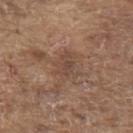<tbp_lesion>
<biopsy_status>not biopsied; imaged during a skin examination</biopsy_status>
<site>upper back</site>
<automated_metrics>
  <border_irregularity_0_10>5.0</border_irregularity_0_10>
  <color_variation_0_10>2.5</color_variation_0_10>
  <peripheral_color_asymmetry>0.5</peripheral_color_asymmetry>
</automated_metrics>
<lesion_size>
  <long_diameter_mm_approx>3.5</long_diameter_mm_approx>
</lesion_size>
<patient>
  <sex>male</sex>
  <age_approx>80</age_approx>
</patient>
<lighting>white-light</lighting>
<image>
  <source>total-body photography crop</source>
  <field_of_view_mm>15</field_of_view_mm>
</image>
</tbp_lesion>